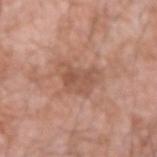Impression: Imaged during a routine full-body skin examination; the lesion was not biopsied and no histopathology is available. Image and clinical context: A region of skin cropped from a whole-body photographic capture, roughly 15 mm wide. Measured at roughly 4.5 mm in maximum diameter. The lesion is on the right forearm. A male subject, aged approximately 60.